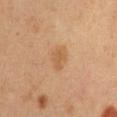Assessment: Part of a total-body skin-imaging series; this lesion was reviewed on a skin check and was not flagged for biopsy. Image and clinical context: From the abdomen. A 15 mm close-up extracted from a 3D total-body photography capture. The recorded lesion diameter is about 3.5 mm. A female subject, about 40 years old. The tile uses cross-polarized illumination.